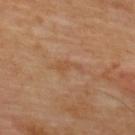Recorded during total-body skin imaging; not selected for excision or biopsy.
Measured at roughly 3.5 mm in maximum diameter.
Located on the upper back.
This image is a 15 mm lesion crop taken from a total-body photograph.
The lesion-visualizer software estimated an area of roughly 3.5 mm², a shape eccentricity near 0.95, and a shape-asymmetry score of about 0.6 (0 = symmetric). The analysis additionally found a lesion-to-skin contrast of about 4.5 (normalized; higher = more distinct).